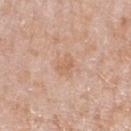The total-body-photography lesion software estimated a lesion area of about 3.5 mm² and a shape eccentricity near 0.65. It also reported a lesion–skin lightness drop of about 7. And it measured a classifier nevus-likeness of about 0/100.
From the arm.
A female patient aged 58 to 62.
The tile uses white-light illumination.
Cropped from a total-body skin-imaging series; the visible field is about 15 mm.